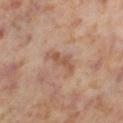follow-up: imaged on a skin check; not biopsied.